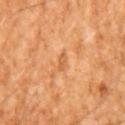Clinical impression:
The lesion was photographed on a routine skin check and not biopsied; there is no pathology result.
Image and clinical context:
On the mid back. Cropped from a total-body skin-imaging series; the visible field is about 15 mm. The patient is a male about 65 years old.